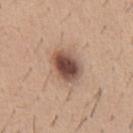{
  "site": "abdomen",
  "patient": {
    "sex": "male",
    "age_approx": 40
  },
  "image": {
    "source": "total-body photography crop",
    "field_of_view_mm": 15
  }
}